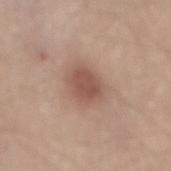<lesion>
  <biopsy_status>not biopsied; imaged during a skin examination</biopsy_status>
</lesion>